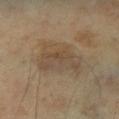Clinical impression: This lesion was catalogued during total-body skin photography and was not selected for biopsy. Acquisition and patient details: Located on the left lower leg. Longest diameter approximately 6 mm. Captured under cross-polarized illumination. A male patient roughly 65 years of age. A 15 mm close-up extracted from a 3D total-body photography capture.